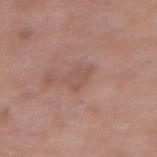Clinical summary: The subject is a female aged 63 to 67. On the abdomen. Automated image analysis of the tile measured a nevus-likeness score of about 0/100 and lesion-presence confidence of about 100/100. Captured under white-light illumination. The lesion's longest dimension is about 3 mm. A lesion tile, about 15 mm wide, cut from a 3D total-body photograph.